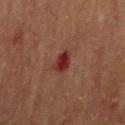This lesion was catalogued during total-body skin photography and was not selected for biopsy. Imaged with cross-polarized lighting. The lesion's longest dimension is about 3 mm. The lesion is on the arm. Cropped from a whole-body photographic skin survey; the tile spans about 15 mm. A female patient roughly 70 years of age. Automated image analysis of the tile measured a lesion–skin lightness drop of about 10 and a lesion-to-skin contrast of about 10.5 (normalized; higher = more distinct). And it measured a within-lesion color-variation index near 2.5/10 and peripheral color asymmetry of about 1. The analysis additionally found a classifier nevus-likeness of about 0/100.